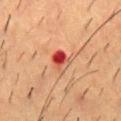The lesion was tiled from a total-body skin photograph and was not biopsied. A male subject aged approximately 55. Imaged with cross-polarized lighting. A close-up tile cropped from a whole-body skin photograph, about 15 mm across. Automated image analysis of the tile measured about 13 CIELAB-L* units darker than the surrounding skin and a lesion-to-skin contrast of about 10 (normalized; higher = more distinct). The software also gave a classifier nevus-likeness of about 0/100 and lesion-presence confidence of about 100/100. The lesion's longest dimension is about 2.5 mm. Located on the mid back.